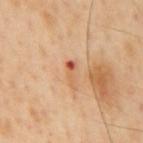biopsy status: no biopsy performed (imaged during a skin exam) | patient: male, in their mid-50s | lesion size: about 3 mm | TBP lesion metrics: a normalized lesion–skin contrast near 7 | lighting: cross-polarized illumination | imaging modality: ~15 mm crop, total-body skin-cancer survey | anatomic site: the mid back.